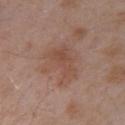* workup — imaged on a skin check; not biopsied
* subject — male, about 55 years old
* image-analysis metrics — an automated nevus-likeness rating near 0 out of 100 and a lesion-detection confidence of about 100/100
* size — ≈5.5 mm
* location — the arm
* acquisition — ~15 mm crop, total-body skin-cancer survey